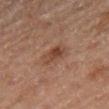Automated image analysis of the tile measured an average lesion color of about L≈37 a*≈19 b*≈25 (CIELAB) and a lesion–skin lightness drop of about 8. The analysis additionally found a color-variation rating of about 4/10 and peripheral color asymmetry of about 1.5. Imaged with cross-polarized lighting. On the right thigh. Cropped from a total-body skin-imaging series; the visible field is about 15 mm. About 3 mm across. A female patient roughly 55 years of age.